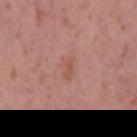Notes:
– notes: no biopsy performed (imaged during a skin exam)